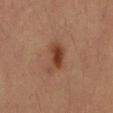<tbp_lesion>
<image>
  <source>total-body photography crop</source>
  <field_of_view_mm>15</field_of_view_mm>
</image>
<automated_metrics>
  <nevus_likeness_0_100>95</nevus_likeness_0_100>
  <lesion_detection_confidence_0_100>100</lesion_detection_confidence_0_100>
</automated_metrics>
<patient>
  <sex>male</sex>
  <age_approx>55</age_approx>
</patient>
<site>abdomen</site>
<lesion_size>
  <long_diameter_mm_approx>4.5</long_diameter_mm_approx>
</lesion_size>
<lighting>cross-polarized</lighting>
</tbp_lesion>